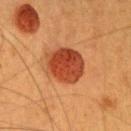Impression:
The lesion was photographed on a routine skin check and not biopsied; there is no pathology result.
Background:
A female patient aged 38–42. A 15 mm close-up extracted from a 3D total-body photography capture. About 5 mm across. The lesion is on the head or neck.